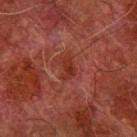This is a cross-polarized tile.
A male subject aged approximately 80.
Automated image analysis of the tile measured a mean CIELAB color near L≈25 a*≈25 b*≈25, about 6 CIELAB-L* units darker than the surrounding skin, and a normalized lesion–skin contrast near 7. The analysis additionally found border irregularity of about 4 on a 0–10 scale, a color-variation rating of about 2/10, and a peripheral color-asymmetry measure near 0.5. And it measured an automated nevus-likeness rating near 0 out of 100 and lesion-presence confidence of about 100/100.
The lesion is on the arm.
The recorded lesion diameter is about 2.5 mm.
This image is a 15 mm lesion crop taken from a total-body photograph.